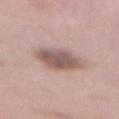{
  "biopsy_status": "not biopsied; imaged during a skin examination",
  "site": "abdomen",
  "image": {
    "source": "total-body photography crop",
    "field_of_view_mm": 15
  },
  "patient": {
    "sex": "female",
    "age_approx": 40
  }
}